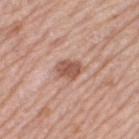The lesion was photographed on a routine skin check and not biopsied; there is no pathology result.
Cropped from a whole-body photographic skin survey; the tile spans about 15 mm.
Captured under white-light illumination.
A female patient aged 63 to 67.
The lesion is located on the right thigh.